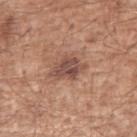Part of a total-body skin-imaging series; this lesion was reviewed on a skin check and was not flagged for biopsy.
A region of skin cropped from a whole-body photographic capture, roughly 15 mm wide.
A male patient aged approximately 65.
From the left upper arm.